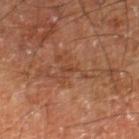  biopsy_status: not biopsied; imaged during a skin examination